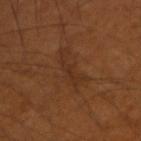{"lesion_size": {"long_diameter_mm_approx": 5.0}, "lighting": "cross-polarized", "image": {"source": "total-body photography crop", "field_of_view_mm": 15}, "patient": {"sex": "male", "age_approx": 55}, "site": "arm", "automated_metrics": {"area_mm2_approx": 7.0, "eccentricity": 0.95, "shape_asymmetry": 0.5, "vs_skin_darker_L": 5.0, "border_irregularity_0_10": 7.5, "color_variation_0_10": 1.0, "peripheral_color_asymmetry": 0.5, "nevus_likeness_0_100": 0, "lesion_detection_confidence_0_100": 95}}